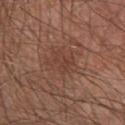Background:
Located on the chest. Longest diameter approximately 3.5 mm. A male patient about 65 years old. A roughly 15 mm field-of-view crop from a total-body skin photograph.